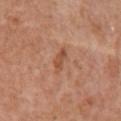Assessment: Captured during whole-body skin photography for melanoma surveillance; the lesion was not biopsied. Image and clinical context: A lesion tile, about 15 mm wide, cut from a 3D total-body photograph. The tile uses white-light illumination. The lesion's longest dimension is about 3.5 mm. The total-body-photography lesion software estimated a footprint of about 3.5 mm², an eccentricity of roughly 0.9, and a shape-asymmetry score of about 0.3 (0 = symmetric). The software also gave a mean CIELAB color near L≈51 a*≈24 b*≈33, roughly 8 lightness units darker than nearby skin, and a normalized border contrast of about 6.5. It also reported a border-irregularity rating of about 3.5/10, internal color variation of about 1 on a 0–10 scale, and radial color variation of about 0.5. The analysis additionally found an automated nevus-likeness rating near 0 out of 100 and lesion-presence confidence of about 100/100. The lesion is located on the front of the torso. A female subject in their 70s.